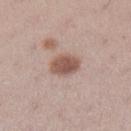Recorded during total-body skin imaging; not selected for excision or biopsy.
Captured under white-light illumination.
A female patient roughly 20 years of age.
Automated tile analysis of the lesion measured a footprint of about 7 mm², an outline eccentricity of about 0.75 (0 = round, 1 = elongated), and two-axis asymmetry of about 0.2. And it measured internal color variation of about 3 on a 0–10 scale and radial color variation of about 1.
A region of skin cropped from a whole-body photographic capture, roughly 15 mm wide.
From the left lower leg.